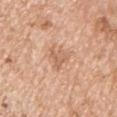Impression:
Part of a total-body skin-imaging series; this lesion was reviewed on a skin check and was not flagged for biopsy.
Background:
On the left upper arm. A region of skin cropped from a whole-body photographic capture, roughly 15 mm wide. A male subject, aged 63 to 67. Automated image analysis of the tile measured a lesion color around L≈62 a*≈21 b*≈33 in CIELAB, about 8 CIELAB-L* units darker than the surrounding skin, and a normalized border contrast of about 5.5. The software also gave a detector confidence of about 100 out of 100 that the crop contains a lesion.